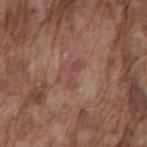anatomic site = the mid back; subject = male, about 75 years old; illumination = white-light; diameter = about 3 mm; image source = ~15 mm crop, total-body skin-cancer survey.